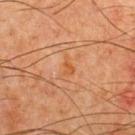Captured during whole-body skin photography for melanoma surveillance; the lesion was not biopsied.
The lesion's longest dimension is about 2.5 mm.
The lesion is on the chest.
Imaged with cross-polarized lighting.
This image is a 15 mm lesion crop taken from a total-body photograph.
A male subject, aged around 65.